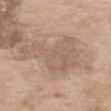The lesion was tiled from a total-body skin photograph and was not biopsied. The lesion is located on the back. A 15 mm close-up extracted from a 3D total-body photography capture. The subject is a female aged 73–77.